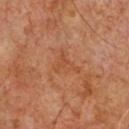This lesion was catalogued during total-body skin photography and was not selected for biopsy.
Captured under cross-polarized illumination.
On the front of the torso.
The total-body-photography lesion software estimated a lesion area of about 6 mm², a shape eccentricity near 0.7, and a symmetry-axis asymmetry near 0.55. The software also gave a lesion color around L≈48 a*≈26 b*≈36 in CIELAB, a lesion–skin lightness drop of about 5, and a normalized lesion–skin contrast near 5. And it measured a border-irregularity rating of about 6/10, internal color variation of about 2 on a 0–10 scale, and radial color variation of about 0.5.
The subject is a male roughly 60 years of age.
A region of skin cropped from a whole-body photographic capture, roughly 15 mm wide.